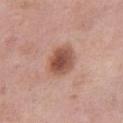notes: total-body-photography surveillance lesion; no biopsy
automated lesion analysis: a footprint of about 10 mm² and an outline eccentricity of about 0.55 (0 = round, 1 = elongated); border irregularity of about 1 on a 0–10 scale, internal color variation of about 5 on a 0–10 scale, and radial color variation of about 1.5; lesion-presence confidence of about 100/100
location: the right thigh
subject: female, approximately 50 years of age
lesion size: ~3.5 mm (longest diameter)
imaging modality: ~15 mm tile from a whole-body skin photo